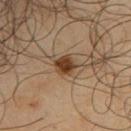| field | value |
|---|---|
| workup | catalogued during a skin exam; not biopsied |
| imaging modality | 15 mm crop, total-body photography |
| automated metrics | roughly 14 lightness units darker than nearby skin; a color-variation rating of about 4.5/10 and peripheral color asymmetry of about 1.5 |
| anatomic site | the upper back |
| patient | male, aged around 65 |
| lesion size | ≈2.5 mm |